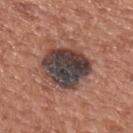{
  "biopsy_status": "not biopsied; imaged during a skin examination",
  "image": {
    "source": "total-body photography crop",
    "field_of_view_mm": 15
  },
  "patient": {
    "sex": "male",
    "age_approx": 55
  },
  "site": "upper back",
  "lesion_size": {
    "long_diameter_mm_approx": 6.0
  },
  "automated_metrics": {
    "area_mm2_approx": 23.0,
    "eccentricity": 0.4,
    "shape_asymmetry": 0.15,
    "cielab_L": 39,
    "cielab_a": 15,
    "cielab_b": 18,
    "vs_skin_darker_L": 16.0,
    "color_variation_0_10": 9.0,
    "peripheral_color_asymmetry": 3.0
  }
}